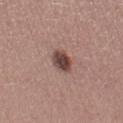Q: Is there a histopathology result?
A: catalogued during a skin exam; not biopsied
Q: What kind of image is this?
A: ~15 mm crop, total-body skin-cancer survey
Q: Patient demographics?
A: female, aged approximately 25
Q: What is the lesion's diameter?
A: ≈3 mm
Q: Where on the body is the lesion?
A: the left thigh